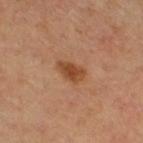The lesion was photographed on a routine skin check and not biopsied; there is no pathology result.
This image is a 15 mm lesion crop taken from a total-body photograph.
The patient is a male approximately 65 years of age.
On the right thigh.